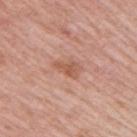notes — total-body-photography surveillance lesion; no biopsy | patient — female, aged 58 to 62 | tile lighting — white-light | image source — 15 mm crop, total-body photography | diameter — ≈3 mm | body site — the right upper arm.